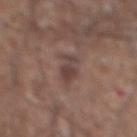Imaged during a routine full-body skin examination; the lesion was not biopsied and no histopathology is available. A male subject roughly 75 years of age. A region of skin cropped from a whole-body photographic capture, roughly 15 mm wide. The lesion is on the front of the torso. Longest diameter approximately 4 mm.